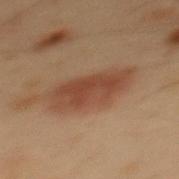Q: Was a biopsy performed?
A: no biopsy performed (imaged during a skin exam)
Q: Lesion location?
A: the mid back
Q: Illumination type?
A: cross-polarized illumination
Q: How large is the lesion?
A: about 6.5 mm
Q: Who is the patient?
A: male, approximately 55 years of age
Q: What kind of image is this?
A: ~15 mm crop, total-body skin-cancer survey